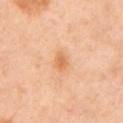Q: Is there a histopathology result?
A: imaged on a skin check; not biopsied
Q: Patient demographics?
A: female, aged 43 to 47
Q: What kind of image is this?
A: ~15 mm crop, total-body skin-cancer survey
Q: Lesion size?
A: ≈2.5 mm
Q: Illumination type?
A: cross-polarized illumination
Q: Lesion location?
A: the left upper arm
Q: Automated lesion metrics?
A: an area of roughly 3.5 mm², a shape eccentricity near 0.75, and two-axis asymmetry of about 0.25; a nevus-likeness score of about 35/100 and a lesion-detection confidence of about 100/100